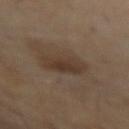{
  "biopsy_status": "not biopsied; imaged during a skin examination",
  "lesion_size": {
    "long_diameter_mm_approx": 4.0
  },
  "patient": {
    "sex": "female",
    "age_approx": 45
  },
  "image": {
    "source": "total-body photography crop",
    "field_of_view_mm": 15
  },
  "lighting": "cross-polarized",
  "site": "arm"
}